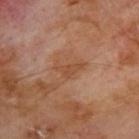{"biopsy_status": "not biopsied; imaged during a skin examination", "automated_metrics": {"eccentricity": 0.85, "peripheral_color_asymmetry": 0.5}, "patient": {"sex": "male", "age_approx": 70}, "site": "upper back", "lesion_size": {"long_diameter_mm_approx": 3.5}, "image": {"source": "total-body photography crop", "field_of_view_mm": 15}}